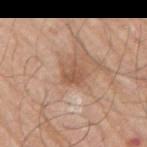notes: catalogued during a skin exam; not biopsied
imaging modality: ~15 mm crop, total-body skin-cancer survey
body site: the arm
size: about 3 mm
tile lighting: white-light illumination
subject: male, aged approximately 65